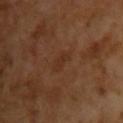The lesion was photographed on a routine skin check and not biopsied; there is no pathology result. The tile uses cross-polarized illumination. About 3 mm across. A male subject about 65 years old. Automated image analysis of the tile measured an outline eccentricity of about 0.9 (0 = round, 1 = elongated) and a symmetry-axis asymmetry near 0.35. It also reported a mean CIELAB color near L≈30 a*≈19 b*≈29, roughly 4 lightness units darker than nearby skin, and a lesion-to-skin contrast of about 5 (normalized; higher = more distinct). The analysis additionally found a border-irregularity rating of about 3.5/10, a within-lesion color-variation index near 1/10, and radial color variation of about 0.5. Cropped from a whole-body photographic skin survey; the tile spans about 15 mm.